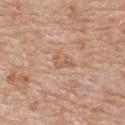biopsy status — no biopsy performed (imaged during a skin exam)
lighting — white-light illumination
body site — the upper back
size — about 2.5 mm
image-analysis metrics — an area of roughly 2.5 mm², an outline eccentricity of about 0.85 (0 = round, 1 = elongated), and two-axis asymmetry of about 0.55
subject — female, roughly 85 years of age or older
imaging modality — ~15 mm crop, total-body skin-cancer survey